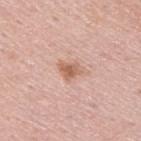Imaged during a routine full-body skin examination; the lesion was not biopsied and no histopathology is available. Cropped from a whole-body photographic skin survey; the tile spans about 15 mm. The patient is a male aged approximately 25. Measured at roughly 2.5 mm in maximum diameter. From the upper back.